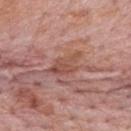Impression:
This lesion was catalogued during total-body skin photography and was not selected for biopsy.
Background:
Located on the back. A region of skin cropped from a whole-body photographic capture, roughly 15 mm wide. A male patient aged approximately 75.